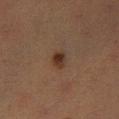Captured during whole-body skin photography for melanoma surveillance; the lesion was not biopsied. Captured under cross-polarized illumination. Longest diameter approximately 2.5 mm. The lesion is located on the left thigh. A 15 mm crop from a total-body photograph taken for skin-cancer surveillance. A female patient approximately 55 years of age.